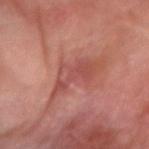Captured during whole-body skin photography for melanoma surveillance; the lesion was not biopsied.
The tile uses cross-polarized illumination.
The patient is a male approximately 70 years of age.
About 5 mm across.
Located on the right forearm.
A 15 mm close-up tile from a total-body photography series done for melanoma screening.